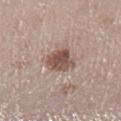Recorded during total-body skin imaging; not selected for excision or biopsy.
A female patient, aged around 50.
The tile uses white-light illumination.
On the left lower leg.
The recorded lesion diameter is about 3.5 mm.
A 15 mm crop from a total-body photograph taken for skin-cancer surveillance.